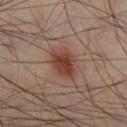biopsy_status: not biopsied; imaged during a skin examination
site: right lower leg
lesion_size:
  long_diameter_mm_approx: 4.5
patient:
  sex: male
  age_approx: 40
lighting: cross-polarized
image:
  source: total-body photography crop
  field_of_view_mm: 15